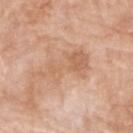{
  "biopsy_status": "not biopsied; imaged during a skin examination",
  "image": {
    "source": "total-body photography crop",
    "field_of_view_mm": 15
  },
  "lesion_size": {
    "long_diameter_mm_approx": 7.0
  },
  "site": "head or neck",
  "lighting": "white-light",
  "patient": {
    "sex": "male",
    "age_approx": 80
  }
}